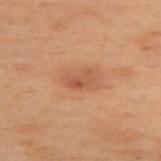  biopsy_status: not biopsied; imaged during a skin examination
  lighting: cross-polarized
  image:
    source: total-body photography crop
    field_of_view_mm: 15
  patient:
    sex: female
    age_approx: 55
  site: upper back